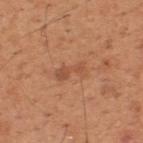Q: Is there a histopathology result?
A: catalogued during a skin exam; not biopsied
Q: What did automated image analysis measure?
A: a mean CIELAB color near L≈52 a*≈24 b*≈34, about 7 CIELAB-L* units darker than the surrounding skin, and a normalized border contrast of about 5
Q: Illumination type?
A: white-light illumination
Q: What kind of image is this?
A: total-body-photography crop, ~15 mm field of view
Q: Lesion location?
A: the upper back
Q: Who is the patient?
A: male, in their mid-50s